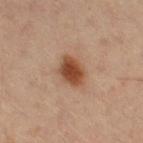Assessment: This lesion was catalogued during total-body skin photography and was not selected for biopsy. Context: The total-body-photography lesion software estimated an area of roughly 8.5 mm², an outline eccentricity of about 0.65 (0 = round, 1 = elongated), and two-axis asymmetry of about 0.15. And it measured a lesion color around L≈49 a*≈23 b*≈34 in CIELAB, about 14 CIELAB-L* units darker than the surrounding skin, and a lesion-to-skin contrast of about 10.5 (normalized; higher = more distinct). And it measured a border-irregularity rating of about 1.5/10, a color-variation rating of about 4.5/10, and a peripheral color-asymmetry measure near 1. It also reported an automated nevus-likeness rating near 100 out of 100. The tile uses cross-polarized illumination. Measured at roughly 4 mm in maximum diameter. A female patient, aged around 40. A lesion tile, about 15 mm wide, cut from a 3D total-body photograph. From the left leg.